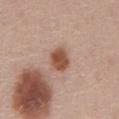| feature | finding |
|---|---|
| acquisition | 15 mm crop, total-body photography |
| site | the abdomen |
| subject | male, about 60 years old |